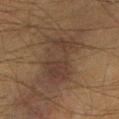* workup — catalogued during a skin exam; not biopsied
* imaging modality — ~15 mm tile from a whole-body skin photo
* illumination — cross-polarized
* anatomic site — the left lower leg
* TBP lesion metrics — a lesion area of about 20 mm², a shape eccentricity near 0.75, and two-axis asymmetry of about 0.25; a lesion color around L≈29 a*≈13 b*≈20 in CIELAB, roughly 5 lightness units darker than nearby skin, and a normalized border contrast of about 6.5
* subject — male, aged approximately 50